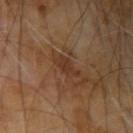Q: Is there a histopathology result?
A: no biopsy performed (imaged during a skin exam)
Q: What is the anatomic site?
A: the right upper arm
Q: What is the imaging modality?
A: ~15 mm crop, total-body skin-cancer survey
Q: Illumination type?
A: cross-polarized
Q: Lesion size?
A: about 3.5 mm
Q: What are the patient's age and sex?
A: male, in their mid-60s